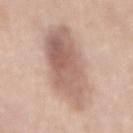Q: Was this lesion biopsied?
A: total-body-photography surveillance lesion; no biopsy
Q: What is the anatomic site?
A: the mid back
Q: What is the lesion's diameter?
A: about 10.5 mm
Q: Automated lesion metrics?
A: a lesion area of about 35 mm², a shape eccentricity near 0.9, and a symmetry-axis asymmetry near 0.15; internal color variation of about 6 on a 0–10 scale and peripheral color asymmetry of about 2; a nevus-likeness score of about 15/100 and a detector confidence of about 100 out of 100 that the crop contains a lesion
Q: Illumination type?
A: white-light
Q: What is the imaging modality?
A: total-body-photography crop, ~15 mm field of view
Q: What are the patient's age and sex?
A: female, roughly 40 years of age